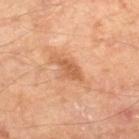This lesion was catalogued during total-body skin photography and was not selected for biopsy.
A lesion tile, about 15 mm wide, cut from a 3D total-body photograph.
The lesion is located on the left leg.
A male subject about 50 years old.
The lesion-visualizer software estimated an eccentricity of roughly 0.85 and a symmetry-axis asymmetry near 0.25. It also reported a border-irregularity index near 3.5/10, internal color variation of about 1.5 on a 0–10 scale, and radial color variation of about 0.5.
Approximately 3.5 mm at its widest.